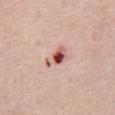• workup · catalogued during a skin exam; not biopsied
• patient · male, aged approximately 65
• anatomic site · the abdomen
• image · 15 mm crop, total-body photography